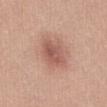A female subject in their 30s. Imaged with white-light lighting. Automated image analysis of the tile measured a lesion area of about 9 mm², a shape eccentricity near 0.7, and two-axis asymmetry of about 0.2. The analysis additionally found a classifier nevus-likeness of about 65/100. The lesion's longest dimension is about 4 mm. Located on the front of the torso. A 15 mm close-up extracted from a 3D total-body photography capture.